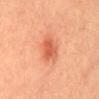Clinical impression:
Recorded during total-body skin imaging; not selected for excision or biopsy.
Background:
Imaged with cross-polarized lighting. Approximately 3.5 mm at its widest. A roughly 15 mm field-of-view crop from a total-body skin photograph. The lesion is located on the abdomen. A female patient, aged around 30.